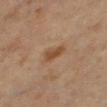Q: Was this lesion biopsied?
A: catalogued during a skin exam; not biopsied
Q: What lighting was used for the tile?
A: cross-polarized
Q: Automated lesion metrics?
A: a footprint of about 4.5 mm², a shape eccentricity near 0.85, and two-axis asymmetry of about 0.25
Q: How large is the lesion?
A: about 3.5 mm
Q: What is the anatomic site?
A: the right thigh
Q: What kind of image is this?
A: total-body-photography crop, ~15 mm field of view
Q: Patient demographics?
A: male, approximately 60 years of age